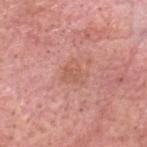No biopsy was performed on this lesion — it was imaged during a full skin examination and was not determined to be concerning. The tile uses white-light illumination. A male patient aged around 60. This image is a 15 mm lesion crop taken from a total-body photograph. Approximately 2.5 mm at its widest. The lesion is on the head or neck.